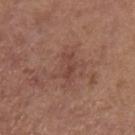biopsy status: imaged on a skin check; not biopsied
tile lighting: white-light
diameter: ~3.5 mm (longest diameter)
imaging modality: total-body-photography crop, ~15 mm field of view
image-analysis metrics: a classifier nevus-likeness of about 0/100 and lesion-presence confidence of about 100/100
patient: female, approximately 65 years of age
anatomic site: the left upper arm